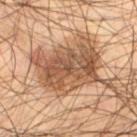Impression: Imaged during a routine full-body skin examination; the lesion was not biopsied and no histopathology is available. Image and clinical context: The tile uses cross-polarized illumination. On the left thigh. The patient is a male aged around 55. A 15 mm close-up tile from a total-body photography series done for melanoma screening. Automated tile analysis of the lesion measured an area of roughly 28 mm², an eccentricity of roughly 0.75, and a symmetry-axis asymmetry near 0.25. And it measured a border-irregularity index near 4.5/10. And it measured a lesion-detection confidence of about 100/100.